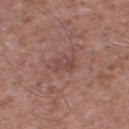<tbp_lesion>
<biopsy_status>not biopsied; imaged during a skin examination</biopsy_status>
</tbp_lesion>